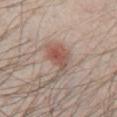Impression: Part of a total-body skin-imaging series; this lesion was reviewed on a skin check and was not flagged for biopsy. Context: A lesion tile, about 15 mm wide, cut from a 3D total-body photograph. The total-body-photography lesion software estimated a mean CIELAB color near L≈54 a*≈19 b*≈25 and about 9 CIELAB-L* units darker than the surrounding skin. A male patient, aged around 40. The lesion is located on the front of the torso. The lesion's longest dimension is about 5 mm. Imaged with white-light lighting.